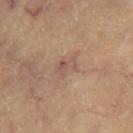No biopsy was performed on this lesion — it was imaged during a full skin examination and was not determined to be concerning.
The recorded lesion diameter is about 2.5 mm.
A roughly 15 mm field-of-view crop from a total-body skin photograph.
A female patient, aged approximately 65.
The lesion is located on the right thigh.
The tile uses cross-polarized illumination.